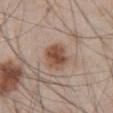notes: total-body-photography surveillance lesion; no biopsy | patient: male, aged 43 to 47 | site: the abdomen | image: 15 mm crop, total-body photography.